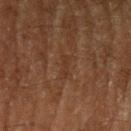Impression: This lesion was catalogued during total-body skin photography and was not selected for biopsy. Context: The lesion's longest dimension is about 2.5 mm. A male subject, roughly 65 years of age. Cropped from a total-body skin-imaging series; the visible field is about 15 mm. The lesion is on the left upper arm.